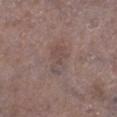{"biopsy_status": "not biopsied; imaged during a skin examination", "image": {"source": "total-body photography crop", "field_of_view_mm": 15}, "lesion_size": {"long_diameter_mm_approx": 4.0}, "site": "right lower leg", "patient": {"sex": "male", "age_approx": 70}}